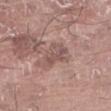Q: Was this lesion biopsied?
A: total-body-photography surveillance lesion; no biopsy